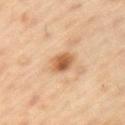Assessment:
Imaged during a routine full-body skin examination; the lesion was not biopsied and no histopathology is available.
Context:
The subject is a male aged approximately 55. From the arm. The lesion-visualizer software estimated an area of roughly 5.5 mm², an outline eccentricity of about 0.5 (0 = round, 1 = elongated), and a shape-asymmetry score of about 0.15 (0 = symmetric). It also reported an average lesion color of about L≈58 a*≈21 b*≈37 (CIELAB), roughly 13 lightness units darker than nearby skin, and a lesion-to-skin contrast of about 9 (normalized; higher = more distinct). And it measured a detector confidence of about 100 out of 100 that the crop contains a lesion. Cropped from a total-body skin-imaging series; the visible field is about 15 mm. The lesion's longest dimension is about 3 mm. The tile uses cross-polarized illumination.